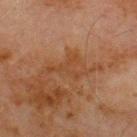Q: Was this lesion biopsied?
A: catalogued during a skin exam; not biopsied
Q: How was this image acquired?
A: total-body-photography crop, ~15 mm field of view
Q: Automated lesion metrics?
A: an outline eccentricity of about 0.9 (0 = round, 1 = elongated); a mean CIELAB color near L≈37 a*≈19 b*≈30, about 5 CIELAB-L* units darker than the surrounding skin, and a lesion-to-skin contrast of about 5 (normalized; higher = more distinct); a border-irregularity index near 9/10, a within-lesion color-variation index near 2.5/10, and radial color variation of about 1; a classifier nevus-likeness of about 0/100
Q: Where on the body is the lesion?
A: the chest
Q: Lesion size?
A: ≈6.5 mm
Q: Who is the patient?
A: male, aged approximately 70